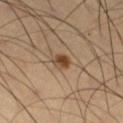workup = no biopsy performed (imaged during a skin exam)
anatomic site = the right thigh
illumination = cross-polarized illumination
patient = male, aged 63–67
acquisition = ~15 mm tile from a whole-body skin photo